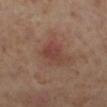Impression:
Captured during whole-body skin photography for melanoma surveillance; the lesion was not biopsied.
Background:
The lesion is on the left lower leg. The tile uses cross-polarized illumination. A region of skin cropped from a whole-body photographic capture, roughly 15 mm wide. About 3 mm across. A female patient roughly 55 years of age.